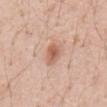Notes:
• follow-up: total-body-photography surveillance lesion; no biopsy